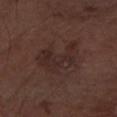Captured during whole-body skin photography for melanoma surveillance; the lesion was not biopsied. From the left forearm. The tile uses white-light illumination. A roughly 15 mm field-of-view crop from a total-body skin photograph. A male subject aged approximately 75. About 5.5 mm across.